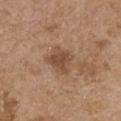  site: chest
  patient:
    sex: female
    age_approx: 65
  automated_metrics:
    area_mm2_approx: 6.5
    eccentricity: 0.5
    shape_asymmetry: 0.25
    cielab_L: 47
    cielab_a: 19
    cielab_b: 30
    vs_skin_darker_L: 10.0
    vs_skin_contrast_norm: 7.5
    lesion_detection_confidence_0_100: 100
  image:
    source: total-body photography crop
    field_of_view_mm: 15